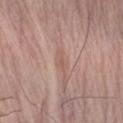Assessment:
This lesion was catalogued during total-body skin photography and was not selected for biopsy.
Clinical summary:
Imaged with white-light lighting. Automated tile analysis of the lesion measured an area of roughly 2.5 mm², an eccentricity of roughly 0.9, and two-axis asymmetry of about 0.35. The analysis additionally found an average lesion color of about L≈57 a*≈20 b*≈25 (CIELAB), a lesion–skin lightness drop of about 7, and a normalized border contrast of about 5. And it measured a border-irregularity index near 4/10, a within-lesion color-variation index near 0/10, and a peripheral color-asymmetry measure near 0. A male subject, roughly 75 years of age. From the arm. A 15 mm close-up tile from a total-body photography series done for melanoma screening.